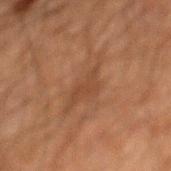The lesion was tiled from a total-body skin photograph and was not biopsied. Cropped from a whole-body photographic skin survey; the tile spans about 15 mm. The subject is a male in their 60s. The total-body-photography lesion software estimated an area of roughly 10 mm², an outline eccentricity of about 0.9 (0 = round, 1 = elongated), and two-axis asymmetry of about 0.45. It also reported a normalized border contrast of about 4.5. From the mid back. The tile uses cross-polarized illumination.